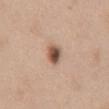Cropped from a whole-body photographic skin survey; the tile spans about 15 mm.
On the abdomen.
A female patient, aged 38 to 42.
An algorithmic analysis of the crop reported a mean CIELAB color near L≈53 a*≈19 b*≈28, roughly 16 lightness units darker than nearby skin, and a lesion-to-skin contrast of about 10.5 (normalized; higher = more distinct). And it measured a border-irregularity rating of about 2/10, a color-variation rating of about 6.5/10, and peripheral color asymmetry of about 2.
Captured under white-light illumination.